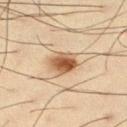No biopsy was performed on this lesion — it was imaged during a full skin examination and was not determined to be concerning.
A region of skin cropped from a whole-body photographic capture, roughly 15 mm wide.
On the leg.
Automated tile analysis of the lesion measured a footprint of about 6 mm², an outline eccentricity of about 0.5 (0 = round, 1 = elongated), and two-axis asymmetry of about 0.2. It also reported a classifier nevus-likeness of about 100/100.
The lesion's longest dimension is about 3 mm.
Imaged with cross-polarized lighting.
A male patient aged around 35.